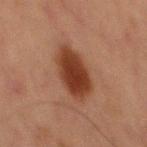| feature | finding |
|---|---|
| biopsy status | no biopsy performed (imaged during a skin exam) |
| lighting | cross-polarized illumination |
| diameter | ~6 mm (longest diameter) |
| imaging modality | ~15 mm crop, total-body skin-cancer survey |
| body site | the abdomen |
| subject | male, in their mid- to late 60s |